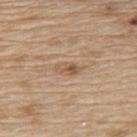Clinical impression:
Part of a total-body skin-imaging series; this lesion was reviewed on a skin check and was not flagged for biopsy.
Background:
Automated image analysis of the tile measured a footprint of about 3.5 mm², an eccentricity of roughly 0.9, and two-axis asymmetry of about 0.3. The software also gave a classifier nevus-likeness of about 5/100 and a lesion-detection confidence of about 100/100. This is a white-light tile. The recorded lesion diameter is about 3 mm. Cropped from a whole-body photographic skin survey; the tile spans about 15 mm. The lesion is on the upper back. A male subject approximately 70 years of age.